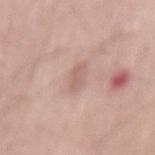No biopsy was performed on this lesion — it was imaged during a full skin examination and was not determined to be concerning. The lesion is on the mid back. A male patient, in their 70s. Captured under white-light illumination. Automated image analysis of the tile measured a footprint of about 4.5 mm², an outline eccentricity of about 0.85 (0 = round, 1 = elongated), and a shape-asymmetry score of about 0.25 (0 = symmetric). It also reported a lesion color around L≈62 a*≈19 b*≈25 in CIELAB and about 7 CIELAB-L* units darker than the surrounding skin. The lesion's longest dimension is about 3 mm. A 15 mm close-up tile from a total-body photography series done for melanoma screening.